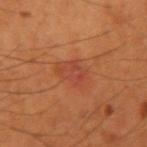biopsy status = total-body-photography surveillance lesion; no biopsy
acquisition = ~15 mm crop, total-body skin-cancer survey
body site = the right upper arm
patient = male, aged 53 to 57